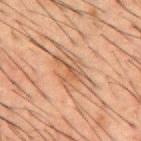Q: Was this lesion biopsied?
A: no biopsy performed (imaged during a skin exam)
Q: Automated lesion metrics?
A: an outline eccentricity of about 0.65 (0 = round, 1 = elongated) and a symmetry-axis asymmetry near 0.5; border irregularity of about 7 on a 0–10 scale, internal color variation of about 4.5 on a 0–10 scale, and peripheral color asymmetry of about 1.5
Q: Lesion size?
A: ≈3.5 mm
Q: Illumination type?
A: cross-polarized illumination
Q: Who is the patient?
A: male, roughly 50 years of age
Q: Where on the body is the lesion?
A: the mid back
Q: What kind of image is this?
A: ~15 mm crop, total-body skin-cancer survey